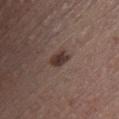biopsy status: catalogued during a skin exam; not biopsied
location: the front of the torso
TBP lesion metrics: a footprint of about 3.5 mm², an outline eccentricity of about 0.75 (0 = round, 1 = elongated), and a shape-asymmetry score of about 0.25 (0 = symmetric); a mean CIELAB color near L≈34 a*≈16 b*≈20 and a normalized lesion–skin contrast near 10; a classifier nevus-likeness of about 95/100 and a lesion-detection confidence of about 100/100
image source: ~15 mm crop, total-body skin-cancer survey
size: ~2.5 mm (longest diameter)
patient: male, aged around 40